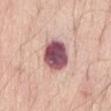The lesion was tiled from a total-body skin photograph and was not biopsied. A lesion tile, about 15 mm wide, cut from a 3D total-body photograph. Captured under white-light illumination. Measured at roughly 4.5 mm in maximum diameter. The lesion is located on the front of the torso. A male subject aged 78–82.